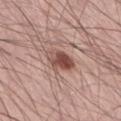workup: catalogued during a skin exam; not biopsied | tile lighting: white-light illumination | patient: male, aged around 55 | anatomic site: the right thigh | acquisition: ~15 mm tile from a whole-body skin photo | diameter: ≈3 mm.